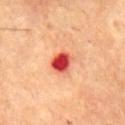The lesion was tiled from a total-body skin photograph and was not biopsied. Captured under cross-polarized illumination. The subject is a male aged around 65. The lesion is on the back. A lesion tile, about 15 mm wide, cut from a 3D total-body photograph.